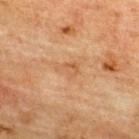{
  "biopsy_status": "not biopsied; imaged during a skin examination",
  "site": "back",
  "automated_metrics": {
    "area_mm2_approx": 3.5,
    "eccentricity": 0.75,
    "shape_asymmetry": 0.6,
    "color_variation_0_10": 3.0,
    "peripheral_color_asymmetry": 1.0
  },
  "lighting": "cross-polarized",
  "lesion_size": {
    "long_diameter_mm_approx": 3.0
  },
  "image": {
    "source": "total-body photography crop",
    "field_of_view_mm": 15
  },
  "patient": {
    "sex": "male",
    "age_approx": 75
  }
}